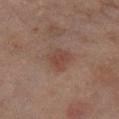Findings:
* notes: catalogued during a skin exam; not biopsied